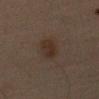Assessment:
This lesion was catalogued during total-body skin photography and was not selected for biopsy.
Clinical summary:
This is a cross-polarized tile. A male patient, in their mid-60s. Automated tile analysis of the lesion measured a footprint of about 6.5 mm², an outline eccentricity of about 0.75 (0 = round, 1 = elongated), and a symmetry-axis asymmetry near 0.15. The software also gave a border-irregularity index near 2/10, a within-lesion color-variation index near 3/10, and peripheral color asymmetry of about 1. On the left upper arm. The lesion's longest dimension is about 3.5 mm. Cropped from a total-body skin-imaging series; the visible field is about 15 mm.